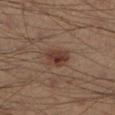follow-up — no biopsy performed (imaged during a skin exam) | patient — male, aged around 40 | diameter — about 2.5 mm | anatomic site — the left lower leg | imaging modality — ~15 mm crop, total-body skin-cancer survey | lighting — cross-polarized illumination.